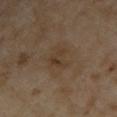Assessment:
Recorded during total-body skin imaging; not selected for excision or biopsy.
Background:
This is a cross-polarized tile. The lesion's longest dimension is about 3.5 mm. A male subject, aged 53–57. A roughly 15 mm field-of-view crop from a total-body skin photograph. From the right upper arm. Automated image analysis of the tile measured lesion-presence confidence of about 100/100.